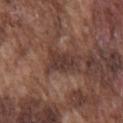biopsy status: catalogued during a skin exam; not biopsied | body site: the chest | diameter: ≈4 mm | acquisition: ~15 mm tile from a whole-body skin photo | illumination: white-light illumination | subject: male, aged 73–77.